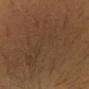No biopsy was performed on this lesion — it was imaged during a full skin examination and was not determined to be concerning. The recorded lesion diameter is about 24.5 mm. An algorithmic analysis of the crop reported a footprint of about 210 mm² and a symmetry-axis asymmetry near 0.25. And it measured an average lesion color of about L≈34 a*≈13 b*≈26 (CIELAB). It also reported a border-irregularity rating of about 5.5/10, a within-lesion color-variation index near 3.5/10, and radial color variation of about 1. A 15 mm close-up tile from a total-body photography series done for melanoma screening. This is a cross-polarized tile. A female subject in their 20s. The lesion is located on the chest.